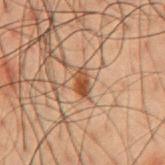Q: Where on the body is the lesion?
A: the back
Q: What is the lesion's diameter?
A: ~3 mm (longest diameter)
Q: Illumination type?
A: cross-polarized illumination
Q: Patient demographics?
A: male, in their 50s
Q: What is the imaging modality?
A: total-body-photography crop, ~15 mm field of view
Q: What did automated image analysis measure?
A: an outline eccentricity of about 0.85 (0 = round, 1 = elongated) and a symmetry-axis asymmetry near 0.2; a lesion color around L≈37 a*≈18 b*≈28 in CIELAB and a lesion–skin lightness drop of about 10; border irregularity of about 2.5 on a 0–10 scale and radial color variation of about 2